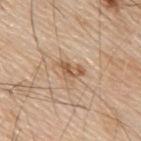Case summary:
* follow-up: total-body-photography surveillance lesion; no biopsy
* lighting: white-light illumination
* anatomic site: the mid back
* lesion diameter: ≈3.5 mm
* image source: 15 mm crop, total-body photography
* image-analysis metrics: internal color variation of about 3.5 on a 0–10 scale and radial color variation of about 1.5; a classifier nevus-likeness of about 30/100 and a lesion-detection confidence of about 100/100
* subject: male, aged approximately 80